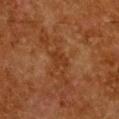The lesion was photographed on a routine skin check and not biopsied; there is no pathology result. Captured under cross-polarized illumination. A female patient, in their 50s. A 15 mm crop from a total-body photograph taken for skin-cancer surveillance. Longest diameter approximately 2.5 mm. Located on the chest. The lesion-visualizer software estimated an outline eccentricity of about 0.7 (0 = round, 1 = elongated). It also reported border irregularity of about 3 on a 0–10 scale, a color-variation rating of about 1/10, and a peripheral color-asymmetry measure near 0.5. And it measured a detector confidence of about 100 out of 100 that the crop contains a lesion.